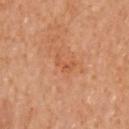Imaged during a routine full-body skin examination; the lesion was not biopsied and no histopathology is available. The lesion is located on the right arm. This is a cross-polarized tile. A lesion tile, about 15 mm wide, cut from a 3D total-body photograph. A female subject aged 58–62.